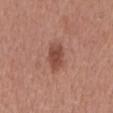Imaged during a routine full-body skin examination; the lesion was not biopsied and no histopathology is available. From the mid back. The total-body-photography lesion software estimated a footprint of about 7 mm², an outline eccentricity of about 0.8 (0 = round, 1 = elongated), and two-axis asymmetry of about 0.15. And it measured a mean CIELAB color near L≈47 a*≈24 b*≈27, a lesion–skin lightness drop of about 11, and a normalized border contrast of about 8. A male subject in their mid-60s. The lesion's longest dimension is about 4 mm. A lesion tile, about 15 mm wide, cut from a 3D total-body photograph. This is a white-light tile.